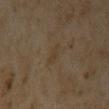The lesion was photographed on a routine skin check and not biopsied; there is no pathology result.
A female subject, approximately 35 years of age.
The lesion is located on the chest.
Cropped from a whole-body photographic skin survey; the tile spans about 15 mm.
This is a cross-polarized tile.
The lesion's longest dimension is about 3.5 mm.